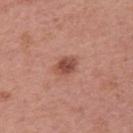Clinical impression: Captured during whole-body skin photography for melanoma surveillance; the lesion was not biopsied. Image and clinical context: Located on the right upper arm. The recorded lesion diameter is about 3 mm. A 15 mm crop from a total-body photograph taken for skin-cancer surveillance. The tile uses white-light illumination. A female patient, roughly 50 years of age.